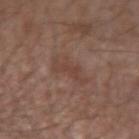• biopsy status: no biopsy performed (imaged during a skin exam)
• patient: male, roughly 75 years of age
• size: about 4 mm
• site: the left forearm
• imaging modality: total-body-photography crop, ~15 mm field of view
• automated metrics: an outline eccentricity of about 0.8 (0 = round, 1 = elongated); a nevus-likeness score of about 0/100 and a lesion-detection confidence of about 100/100
• tile lighting: white-light illumination